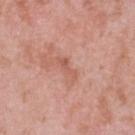No biopsy was performed on this lesion — it was imaged during a full skin examination and was not determined to be concerning. A lesion tile, about 15 mm wide, cut from a 3D total-body photograph. The lesion is located on the upper back. The patient is a female aged 38 to 42.